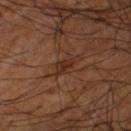The lesion was tiled from a total-body skin photograph and was not biopsied.
A male subject about 60 years old.
On the left thigh.
A 15 mm close-up tile from a total-body photography series done for melanoma screening.